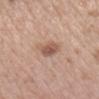Imaged during a routine full-body skin examination; the lesion was not biopsied and no histopathology is available.
Cropped from a whole-body photographic skin survey; the tile spans about 15 mm.
Imaged with white-light lighting.
The lesion's longest dimension is about 3 mm.
A female patient, about 40 years old.
From the head or neck.
The total-body-photography lesion software estimated a footprint of about 5 mm² and an eccentricity of roughly 0.7. It also reported a mean CIELAB color near L≈55 a*≈20 b*≈28, about 11 CIELAB-L* units darker than the surrounding skin, and a lesion-to-skin contrast of about 7.5 (normalized; higher = more distinct). The software also gave an automated nevus-likeness rating near 80 out of 100.